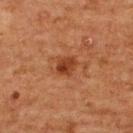The lesion was tiled from a total-body skin photograph and was not biopsied. A female subject, in their 50s. The lesion's longest dimension is about 2.5 mm. Cropped from a total-body skin-imaging series; the visible field is about 15 mm. The lesion is on the upper back. Imaged with cross-polarized lighting.